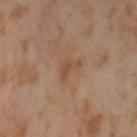{"biopsy_status": "not biopsied; imaged during a skin examination", "site": "left thigh", "lighting": "cross-polarized", "image": {"source": "total-body photography crop", "field_of_view_mm": 15}, "patient": {"sex": "female", "age_approx": 55}, "lesion_size": {"long_diameter_mm_approx": 3.0}}